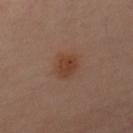| field | value |
|---|---|
| follow-up | total-body-photography surveillance lesion; no biopsy |
| patient | male, in their mid- to late 60s |
| site | the left upper arm |
| lesion diameter | about 3 mm |
| illumination | cross-polarized illumination |
| acquisition | 15 mm crop, total-body photography |